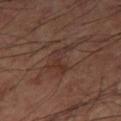Recorded during total-body skin imaging; not selected for excision or biopsy. A male patient, aged 58 to 62. On the leg. A 15 mm crop from a total-body photograph taken for skin-cancer surveillance. About 4 mm across.